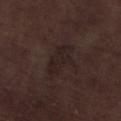follow-up: no biopsy performed (imaged during a skin exam)
tile lighting: white-light illumination
subject: male, aged 68–72
lesion size: about 5 mm
imaging modality: ~15 mm tile from a whole-body skin photo
automated metrics: an eccentricity of roughly 0.85 and a shape-asymmetry score of about 0.3 (0 = symmetric); a lesion color around L≈20 a*≈12 b*≈14 in CIELAB; a border-irregularity index near 4/10, a within-lesion color-variation index near 2/10, and radial color variation of about 0.5; an automated nevus-likeness rating near 0 out of 100 and lesion-presence confidence of about 80/100
anatomic site: the left lower leg